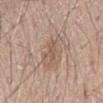Q: Was a biopsy performed?
A: no biopsy performed (imaged during a skin exam)
Q: How was this image acquired?
A: ~15 mm crop, total-body skin-cancer survey
Q: Lesion size?
A: ≈3 mm
Q: Automated lesion metrics?
A: a footprint of about 3.5 mm², an eccentricity of roughly 0.85, and two-axis asymmetry of about 0.35; border irregularity of about 4 on a 0–10 scale, internal color variation of about 1 on a 0–10 scale, and a peripheral color-asymmetry measure near 0.5
Q: Who is the patient?
A: male, roughly 20 years of age
Q: Illumination type?
A: white-light
Q: What is the anatomic site?
A: the front of the torso